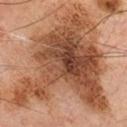automated lesion analysis: an area of roughly 100 mm² and two-axis asymmetry of about 0.5
acquisition: ~15 mm crop, total-body skin-cancer survey
tile lighting: cross-polarized
patient: male, aged 68 to 72
size: about 15 mm
anatomic site: the chest